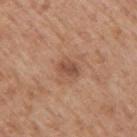Q: Lesion location?
A: the right upper arm
Q: How was this image acquired?
A: total-body-photography crop, ~15 mm field of view
Q: How large is the lesion?
A: about 2.5 mm
Q: Who is the patient?
A: male, roughly 60 years of age